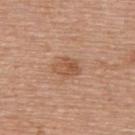  biopsy_status: not biopsied; imaged during a skin examination
  patient:
    sex: female
    age_approx: 60
  lesion_size:
    long_diameter_mm_approx: 3.5
  site: upper back
  lighting: white-light
  image:
    source: total-body photography crop
    field_of_view_mm: 15